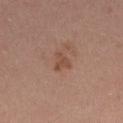Part of a total-body skin-imaging series; this lesion was reviewed on a skin check and was not flagged for biopsy.